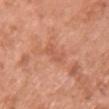Impression: Captured during whole-body skin photography for melanoma surveillance; the lesion was not biopsied. Clinical summary: Located on the chest. The recorded lesion diameter is about 2.5 mm. Captured under white-light illumination. An algorithmic analysis of the crop reported a lesion–skin lightness drop of about 7. The software also gave border irregularity of about 5 on a 0–10 scale and a within-lesion color-variation index near 0/10. It also reported a lesion-detection confidence of about 100/100. A 15 mm crop from a total-body photograph taken for skin-cancer surveillance. A female patient, about 50 years old.